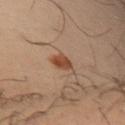Findings:
* biopsy status — imaged on a skin check; not biopsied
* acquisition — ~15 mm crop, total-body skin-cancer survey
* lighting — cross-polarized illumination
* size — about 2.5 mm
* subject — male, aged 38–42
* anatomic site — the arm